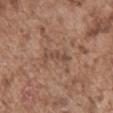The lesion was photographed on a routine skin check and not biopsied; there is no pathology result. An algorithmic analysis of the crop reported a lesion–skin lightness drop of about 7 and a normalized border contrast of about 6. The analysis additionally found an automated nevus-likeness rating near 0 out of 100 and a lesion-detection confidence of about 55/100. A male subject, roughly 75 years of age. About 3.5 mm across. A 15 mm close-up extracted from a 3D total-body photography capture. From the abdomen.